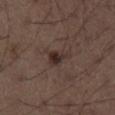Impression: No biopsy was performed on this lesion — it was imaged during a full skin examination and was not determined to be concerning. Clinical summary: The lesion is located on the right thigh. The tile uses white-light illumination. A 15 mm crop from a total-body photograph taken for skin-cancer surveillance. The patient is a male in their 50s. The recorded lesion diameter is about 3 mm. An algorithmic analysis of the crop reported a lesion area of about 4.5 mm² and a shape eccentricity near 0.8. It also reported an automated nevus-likeness rating near 70 out of 100 and a lesion-detection confidence of about 100/100.